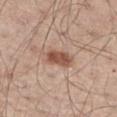No biopsy was performed on this lesion — it was imaged during a full skin examination and was not determined to be concerning. The lesion's longest dimension is about 3.5 mm. A male patient approximately 60 years of age. The tile uses white-light illumination. A 15 mm crop from a total-body photograph taken for skin-cancer surveillance. On the right thigh.